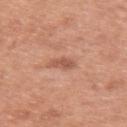The lesion was tiled from a total-body skin photograph and was not biopsied.
A 15 mm close-up tile from a total-body photography series done for melanoma screening.
A female patient, aged around 50.
The recorded lesion diameter is about 3 mm.
Automated image analysis of the tile measured a lesion area of about 3.5 mm², an eccentricity of roughly 0.85, and a shape-asymmetry score of about 0.2 (0 = symmetric). The software also gave border irregularity of about 2.5 on a 0–10 scale, a color-variation rating of about 1.5/10, and radial color variation of about 0.5. It also reported a nevus-likeness score of about 0/100.
The tile uses white-light illumination.
The lesion is on the right upper arm.